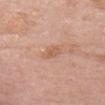patient:
  sex: female
  age_approx: 60
image:
  source: total-body photography crop
  field_of_view_mm: 15
lesion_size:
  long_diameter_mm_approx: 2.5
site: head or neck